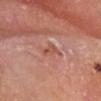notes = no biopsy performed (imaged during a skin exam) | illumination = white-light | subject = male, aged 78–82 | site = the head or neck | image = ~15 mm crop, total-body skin-cancer survey | lesion size = ≈2.5 mm.